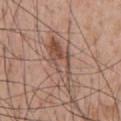The lesion was photographed on a routine skin check and not biopsied; there is no pathology result.
The lesion is on the upper back.
A male subject, in their mid- to late 50s.
The tile uses white-light illumination.
Approximately 7 mm at its widest.
This image is a 15 mm lesion crop taken from a total-body photograph.